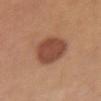{"biopsy_status": "not biopsied; imaged during a skin examination", "lighting": "white-light", "patient": {"sex": "female", "age_approx": 55}, "site": "chest", "image": {"source": "total-body photography crop", "field_of_view_mm": 15}, "automated_metrics": {"vs_skin_darker_L": 13.0, "vs_skin_contrast_norm": 9.5, "border_irregularity_0_10": 1.5, "color_variation_0_10": 2.5, "peripheral_color_asymmetry": 1.0, "lesion_detection_confidence_0_100": 100}, "lesion_size": {"long_diameter_mm_approx": 5.5}}